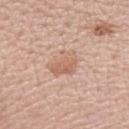This lesion was catalogued during total-body skin photography and was not selected for biopsy.
Automated tile analysis of the lesion measured an average lesion color of about L≈62 a*≈20 b*≈30 (CIELAB), a lesion–skin lightness drop of about 8, and a lesion-to-skin contrast of about 6 (normalized; higher = more distinct).
Cropped from a whole-body photographic skin survey; the tile spans about 15 mm.
The lesion is located on the arm.
Approximately 3.5 mm at its widest.
A female subject about 35 years old.